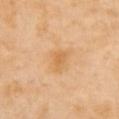Imaged with cross-polarized lighting. A close-up tile cropped from a whole-body skin photograph, about 15 mm across. A female patient, approximately 55 years of age. Measured at roughly 3 mm in maximum diameter. The lesion is on the chest.